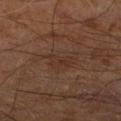workup=no biopsy performed (imaged during a skin exam) | patient=male, aged 58–62 | image-analysis metrics=a lesion area of about 4.5 mm² and a shape eccentricity near 0.8; internal color variation of about 2 on a 0–10 scale and peripheral color asymmetry of about 0.5 | image=~15 mm tile from a whole-body skin photo | tile lighting=cross-polarized illumination | anatomic site=the right leg | lesion diameter=~3 mm (longest diameter).